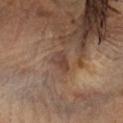Findings:
– biopsy status · no biopsy performed (imaged during a skin exam)
– subject · female, aged around 60
– image source · ~15 mm tile from a whole-body skin photo
– tile lighting · cross-polarized illumination
– lesion size · about 2.5 mm
– body site · the head or neck